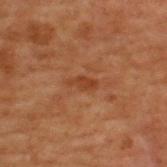Cropped from a total-body skin-imaging series; the visible field is about 15 mm.
The lesion is on the upper back.
Captured under cross-polarized illumination.
A male patient approximately 70 years of age.
The recorded lesion diameter is about 3 mm.